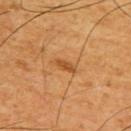Acquisition and patient details:
Automated image analysis of the tile measured an area of roughly 2.5 mm². The software also gave border irregularity of about 3.5 on a 0–10 scale, internal color variation of about 0 on a 0–10 scale, and radial color variation of about 0. The software also gave a classifier nevus-likeness of about 30/100 and a detector confidence of about 100 out of 100 that the crop contains a lesion. On the front of the torso. A roughly 15 mm field-of-view crop from a total-body skin photograph. Imaged with cross-polarized lighting. The lesion's longest dimension is about 3 mm. A male patient, in their 60s.